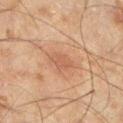Case summary:
– workup: imaged on a skin check; not biopsied
– subject: male, about 45 years old
– lesion size: about 3 mm
– anatomic site: the leg
– imaging modality: total-body-photography crop, ~15 mm field of view